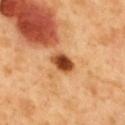Impression:
The lesion was photographed on a routine skin check and not biopsied; there is no pathology result.
Acquisition and patient details:
A 15 mm crop from a total-body photograph taken for skin-cancer surveillance. Automated tile analysis of the lesion measured a lesion area of about 5.5 mm², an outline eccentricity of about 0.75 (0 = round, 1 = elongated), and a shape-asymmetry score of about 0.2 (0 = symmetric). The analysis additionally found a lesion color around L≈48 a*≈28 b*≈41 in CIELAB and a lesion–skin lightness drop of about 20. It also reported border irregularity of about 2 on a 0–10 scale and a color-variation rating of about 5/10. The recorded lesion diameter is about 3 mm. A male subject, aged approximately 50. Captured under cross-polarized illumination. On the mid back.